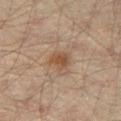| key | value |
|---|---|
| follow-up | imaged on a skin check; not biopsied |
| patient | male, aged 43 to 47 |
| site | the right thigh |
| tile lighting | cross-polarized |
| image source | ~15 mm tile from a whole-body skin photo |
| automated lesion analysis | a lesion color around L≈42 a*≈16 b*≈27 in CIELAB, roughly 8 lightness units darker than nearby skin, and a lesion-to-skin contrast of about 7 (normalized; higher = more distinct); border irregularity of about 1.5 on a 0–10 scale, a color-variation rating of about 2/10, and peripheral color asymmetry of about 0.5 |